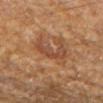{"biopsy_status": "not biopsied; imaged during a skin examination", "image": {"source": "total-body photography crop", "field_of_view_mm": 15}, "lighting": "cross-polarized", "lesion_size": {"long_diameter_mm_approx": 4.0}, "site": "left forearm", "automated_metrics": {"area_mm2_approx": 6.5, "eccentricity": 0.85, "shape_asymmetry": 0.6, "cielab_L": 41, "cielab_a": 20, "cielab_b": 29, "vs_skin_darker_L": 8.0, "vs_skin_contrast_norm": 6.5, "border_irregularity_0_10": 7.5, "color_variation_0_10": 2.0, "peripheral_color_asymmetry": 0.5, "nevus_likeness_0_100": 10}, "patient": {"sex": "male", "age_approx": 65}}